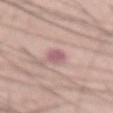Findings:
– notes: imaged on a skin check; not biopsied
– imaging modality: 15 mm crop, total-body photography
– size: about 2.5 mm
– location: the abdomen
– TBP lesion metrics: border irregularity of about 2 on a 0–10 scale and a color-variation rating of about 2/10; a detector confidence of about 95 out of 100 that the crop contains a lesion
– lighting: white-light
– patient: male, aged 58–62